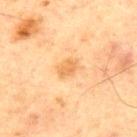Q: What are the patient's age and sex?
A: male, about 65 years old
Q: How was the tile lit?
A: cross-polarized
Q: Lesion location?
A: the front of the torso
Q: How was this image acquired?
A: total-body-photography crop, ~15 mm field of view
Q: What did automated image analysis measure?
A: a shape eccentricity near 0.75; a mean CIELAB color near L≈56 a*≈19 b*≈39, about 8 CIELAB-L* units darker than the surrounding skin, and a normalized border contrast of about 6.5
Q: What is the lesion's diameter?
A: ≈2.5 mm